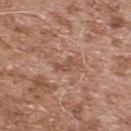Captured during whole-body skin photography for melanoma surveillance; the lesion was not biopsied.
The tile uses white-light illumination.
A male subject aged around 55.
About 2.5 mm across.
From the back.
An algorithmic analysis of the crop reported an eccentricity of roughly 0.85 and a shape-asymmetry score of about 0.5 (0 = symmetric). The analysis additionally found a color-variation rating of about 0/10 and peripheral color asymmetry of about 0. It also reported an automated nevus-likeness rating near 0 out of 100.
A close-up tile cropped from a whole-body skin photograph, about 15 mm across.